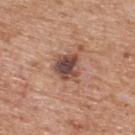  biopsy_status: not biopsied; imaged during a skin examination
  lesion_size:
    long_diameter_mm_approx: 3.5
  automated_metrics:
    area_mm2_approx: 8.5
    shape_asymmetry: 0.25
    lesion_detection_confidence_0_100: 100
  site: back
  lighting: white-light
  image:
    source: total-body photography crop
    field_of_view_mm: 15
  patient:
    sex: male
    age_approx: 60